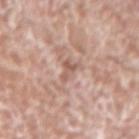Recorded during total-body skin imaging; not selected for excision or biopsy.
Located on the arm.
A lesion tile, about 15 mm wide, cut from a 3D total-body photograph.
A male subject, in their mid-70s.
The tile uses white-light illumination.
An algorithmic analysis of the crop reported an eccentricity of roughly 0.9 and two-axis asymmetry of about 0.6. And it measured a border-irregularity index near 6.5/10, a color-variation rating of about 0/10, and radial color variation of about 0. It also reported an automated nevus-likeness rating near 0 out of 100 and a detector confidence of about 70 out of 100 that the crop contains a lesion.